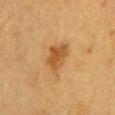Notes:
- notes: catalogued during a skin exam; not biopsied
- patient: female, approximately 40 years of age
- imaging modality: 15 mm crop, total-body photography
- diameter: about 4 mm
- illumination: cross-polarized
- site: the chest